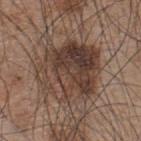site: upper back
automated_metrics:
  area_mm2_approx: 34.0
  eccentricity: 0.35
  cielab_L: 40
  cielab_a: 16
  cielab_b: 23
  vs_skin_darker_L: 11.0
  vs_skin_contrast_norm: 9.0
  nevus_likeness_0_100: 20
  lesion_detection_confidence_0_100: 100
patient:
  sex: male
  age_approx: 45
lighting: white-light
image:
  source: total-body photography crop
  field_of_view_mm: 15